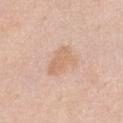Case summary:
– follow-up · catalogued during a skin exam; not biopsied
– subject · female, about 65 years old
– imaging modality · 15 mm crop, total-body photography
– location · the front of the torso
– diameter · about 4 mm